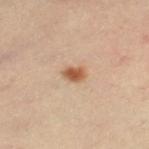Assessment:
Recorded during total-body skin imaging; not selected for excision or biopsy.
Context:
A female patient about 55 years old. A close-up tile cropped from a whole-body skin photograph, about 15 mm across. Captured under cross-polarized illumination. The lesion is on the leg. Automated tile analysis of the lesion measured a footprint of about 4 mm², a shape eccentricity near 0.75, and a shape-asymmetry score of about 0.15 (0 = symmetric). The software also gave roughly 13 lightness units darker than nearby skin and a lesion-to-skin contrast of about 9.5 (normalized; higher = more distinct). And it measured border irregularity of about 1.5 on a 0–10 scale and peripheral color asymmetry of about 1. The analysis additionally found a nevus-likeness score of about 100/100 and a detector confidence of about 100 out of 100 that the crop contains a lesion.